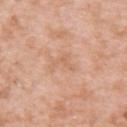Recorded during total-body skin imaging; not selected for excision or biopsy. The tile uses white-light illumination. A roughly 15 mm field-of-view crop from a total-body skin photograph. A female subject approximately 40 years of age. Automated image analysis of the tile measured an outline eccentricity of about 0.85 (0 = round, 1 = elongated) and two-axis asymmetry of about 0.75. The software also gave a mean CIELAB color near L≈64 a*≈22 b*≈34, a lesion–skin lightness drop of about 6, and a normalized border contrast of about 4.5. It also reported a classifier nevus-likeness of about 0/100 and lesion-presence confidence of about 100/100. Located on the arm. Measured at roughly 3.5 mm in maximum diameter.